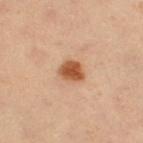This lesion was catalogued during total-body skin photography and was not selected for biopsy.
Measured at roughly 2.5 mm in maximum diameter.
A 15 mm close-up tile from a total-body photography series done for melanoma screening.
A female subject, roughly 35 years of age.
Captured under cross-polarized illumination.
The lesion is on the right thigh.